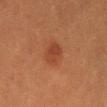From the front of the torso. Captured under cross-polarized illumination. A female patient, aged approximately 30. Automated image analysis of the tile measured an average lesion color of about L≈40 a*≈26 b*≈33 (CIELAB), a lesion–skin lightness drop of about 7, and a normalized border contrast of about 6. The software also gave an automated nevus-likeness rating near 95 out of 100. Cropped from a total-body skin-imaging series; the visible field is about 15 mm.